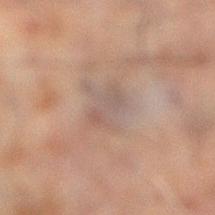Impression: Part of a total-body skin-imaging series; this lesion was reviewed on a skin check and was not flagged for biopsy. Context: A 15 mm close-up tile from a total-body photography series done for melanoma screening. The tile uses cross-polarized illumination. A male subject, aged approximately 60. On the left leg.